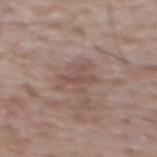Findings:
* workup: no biopsy performed (imaged during a skin exam)
* lesion diameter: ~4.5 mm (longest diameter)
* subject: male, about 70 years old
* imaging modality: 15 mm crop, total-body photography
* body site: the upper back
* illumination: white-light